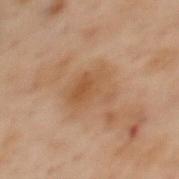Recorded during total-body skin imaging; not selected for excision or biopsy. This image is a 15 mm lesion crop taken from a total-body photograph. The lesion's longest dimension is about 5 mm. The lesion is located on the upper back. An algorithmic analysis of the crop reported a shape eccentricity near 0.75 and two-axis asymmetry of about 0.3. The analysis additionally found a nevus-likeness score of about 5/100 and a detector confidence of about 100 out of 100 that the crop contains a lesion. A female patient, approximately 55 years of age.